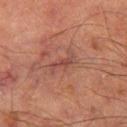Assessment: Imaged during a routine full-body skin examination; the lesion was not biopsied and no histopathology is available. Context: Located on the leg. Cropped from a whole-body photographic skin survey; the tile spans about 15 mm. A male patient, in their mid- to late 60s.